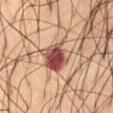biopsy_status: not biopsied; imaged during a skin examination
image:
  source: total-body photography crop
  field_of_view_mm: 15
lighting: cross-polarized
patient:
  sex: male
  age_approx: 60
lesion_size:
  long_diameter_mm_approx: 4.0
site: front of the torso